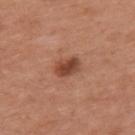No biopsy was performed on this lesion — it was imaged during a full skin examination and was not determined to be concerning. A female patient approximately 40 years of age. Automated image analysis of the tile measured a lesion-to-skin contrast of about 9 (normalized; higher = more distinct). It also reported a border-irregularity rating of about 2/10, a color-variation rating of about 4/10, and radial color variation of about 1. And it measured an automated nevus-likeness rating near 80 out of 100 and a detector confidence of about 100 out of 100 that the crop contains a lesion. Located on the back. A roughly 15 mm field-of-view crop from a total-body skin photograph.